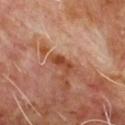Imaged during a routine full-body skin examination; the lesion was not biopsied and no histopathology is available.
The patient is a male aged 63 to 67.
Automated tile analysis of the lesion measured a color-variation rating of about 0/10 and a peripheral color-asymmetry measure near 0. The software also gave a nevus-likeness score of about 0/100 and lesion-presence confidence of about 100/100.
Captured under cross-polarized illumination.
Measured at roughly 2.5 mm in maximum diameter.
The lesion is located on the chest.
This image is a 15 mm lesion crop taken from a total-body photograph.